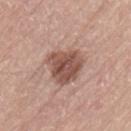notes = total-body-photography surveillance lesion; no biopsy | image-analysis metrics = a shape eccentricity near 0.25 and a symmetry-axis asymmetry near 0.25; an automated nevus-likeness rating near 50 out of 100 and lesion-presence confidence of about 100/100 | body site = the back | patient = male, approximately 60 years of age | lesion size = ~4.5 mm (longest diameter) | acquisition = total-body-photography crop, ~15 mm field of view | tile lighting = white-light.